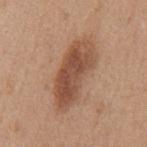This lesion was catalogued during total-body skin photography and was not selected for biopsy.
The subject is a female aged approximately 40.
A close-up tile cropped from a whole-body skin photograph, about 15 mm across.
The lesion is located on the mid back.